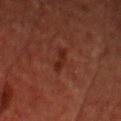biopsy_status: not biopsied; imaged during a skin examination
patient:
  sex: male
  age_approx: 60
site: head or neck
image:
  source: total-body photography crop
  field_of_view_mm: 15